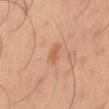notes: total-body-photography surveillance lesion; no biopsy | illumination: cross-polarized | TBP lesion metrics: border irregularity of about 3 on a 0–10 scale and a within-lesion color-variation index near 0/10 | subject: male, aged 48–52 | imaging modality: 15 mm crop, total-body photography | site: the right thigh | lesion diameter: about 2.5 mm.